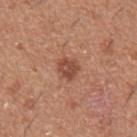follow-up = no biopsy performed (imaged during a skin exam) | image source = total-body-photography crop, ~15 mm field of view | automated lesion analysis = border irregularity of about 2.5 on a 0–10 scale and a color-variation rating of about 2.5/10; an automated nevus-likeness rating near 70 out of 100 | tile lighting = white-light illumination | site = the left forearm | lesion size = ≈2.5 mm | subject = male, aged 38–42.